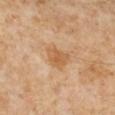Q: Was this lesion biopsied?
A: no biopsy performed (imaged during a skin exam)
Q: What is the anatomic site?
A: the right lower leg
Q: How large is the lesion?
A: ≈3 mm
Q: Patient demographics?
A: female, approximately 50 years of age
Q: How was this image acquired?
A: total-body-photography crop, ~15 mm field of view